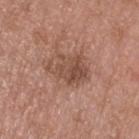<record>
<biopsy_status>not biopsied; imaged during a skin examination</biopsy_status>
<image>
  <source>total-body photography crop</source>
  <field_of_view_mm>15</field_of_view_mm>
</image>
<site>head or neck</site>
<patient>
  <sex>male</sex>
  <age_approx>50</age_approx>
</patient>
</record>